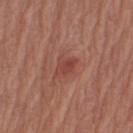<record>
<lesion_size>
  <long_diameter_mm_approx>3.0</long_diameter_mm_approx>
</lesion_size>
<patient>
  <sex>male</sex>
  <age_approx>75</age_approx>
</patient>
<site>right upper arm</site>
<image>
  <source>total-body photography crop</source>
  <field_of_view_mm>15</field_of_view_mm>
</image>
</record>